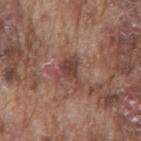Captured during whole-body skin photography for melanoma surveillance; the lesion was not biopsied. A lesion tile, about 15 mm wide, cut from a 3D total-body photograph. Longest diameter approximately 3.5 mm. Automated image analysis of the tile measured a lesion–skin lightness drop of about 10 and a normalized lesion–skin contrast near 8. The software also gave internal color variation of about 3 on a 0–10 scale and a peripheral color-asymmetry measure near 1. Located on the mid back. This is a white-light tile. A male subject in their mid- to late 70s.